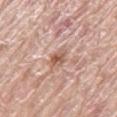Q: Is there a histopathology result?
A: no biopsy performed (imaged during a skin exam)
Q: What lighting was used for the tile?
A: white-light illumination
Q: What kind of image is this?
A: ~15 mm crop, total-body skin-cancer survey
Q: Patient demographics?
A: female, aged approximately 60
Q: Where on the body is the lesion?
A: the right thigh
Q: What is the lesion's diameter?
A: ~2.5 mm (longest diameter)